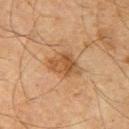The lesion was photographed on a routine skin check and not biopsied; there is no pathology result.
A male patient, approximately 65 years of age.
Automated image analysis of the tile measured an area of roughly 9.5 mm² and a shape-asymmetry score of about 0.3 (0 = symmetric). And it measured a nevus-likeness score of about 20/100 and a detector confidence of about 100 out of 100 that the crop contains a lesion.
A 15 mm close-up tile from a total-body photography series done for melanoma screening.
Located on the arm.
About 4 mm across.